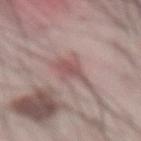Q: Is there a histopathology result?
A: catalogued during a skin exam; not biopsied
Q: Who is the patient?
A: male, aged 68–72
Q: Lesion location?
A: the abdomen
Q: Illumination type?
A: white-light illumination
Q: What is the lesion's diameter?
A: ≈5.5 mm
Q: How was this image acquired?
A: ~15 mm tile from a whole-body skin photo